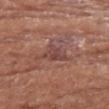<record>
  <biopsy_status>not biopsied; imaged during a skin examination</biopsy_status>
  <patient>
    <sex>female</sex>
    <age_approx>75</age_approx>
  </patient>
  <site>arm</site>
  <image>
    <source>total-body photography crop</source>
    <field_of_view_mm>15</field_of_view_mm>
  </image>
</record>